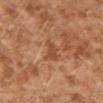  biopsy_status: not biopsied; imaged during a skin examination
  patient:
    sex: male
    age_approx: 30
  lesion_size:
    long_diameter_mm_approx: 3.0
  lighting: cross-polarized
  site: left lower leg
  automated_metrics:
    nevus_likeness_0_100: 0
  image:
    source: total-body photography crop
    field_of_view_mm: 15